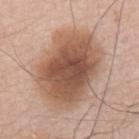Findings:
* biopsy status — total-body-photography surveillance lesion; no biopsy
* acquisition — ~15 mm crop, total-body skin-cancer survey
* diameter — ~9.5 mm (longest diameter)
* location — the back
* tile lighting — white-light
* automated metrics — a lesion color around L≈54 a*≈19 b*≈29 in CIELAB and a normalized border contrast of about 9.5; a nevus-likeness score of about 70/100 and a detector confidence of about 100 out of 100 that the crop contains a lesion
* subject — male, aged around 75